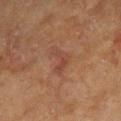• workup · total-body-photography surveillance lesion; no biopsy
• anatomic site · the left forearm
• tile lighting · cross-polarized illumination
• image-analysis metrics · a footprint of about 5 mm², an outline eccentricity of about 0.8 (0 = round, 1 = elongated), and a shape-asymmetry score of about 0.6 (0 = symmetric); a lesion–skin lightness drop of about 5; border irregularity of about 6 on a 0–10 scale, a color-variation rating of about 2/10, and radial color variation of about 0.5; a classifier nevus-likeness of about 0/100 and a lesion-detection confidence of about 100/100
• patient · female, aged around 80
• acquisition · ~15 mm tile from a whole-body skin photo
• lesion diameter · about 3.5 mm